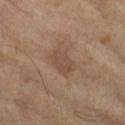{
  "biopsy_status": "not biopsied; imaged during a skin examination",
  "site": "left lower leg",
  "automated_metrics": {
    "cielab_L": 47,
    "cielab_a": 17,
    "cielab_b": 28,
    "vs_skin_contrast_norm": 5.0,
    "nevus_likeness_0_100": 0
  },
  "lighting": "cross-polarized",
  "patient": {
    "sex": "female",
    "age_approx": 75
  },
  "lesion_size": {
    "long_diameter_mm_approx": 3.0
  },
  "image": {
    "source": "total-body photography crop",
    "field_of_view_mm": 15
  }
}